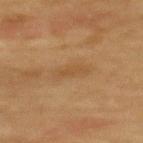automated metrics: a footprint of about 3.5 mm², a shape eccentricity near 0.95, and a shape-asymmetry score of about 0.25 (0 = symmetric); an average lesion color of about L≈43 a*≈16 b*≈33 (CIELAB) and a normalized lesion–skin contrast near 5; a border-irregularity rating of about 3.5/10, internal color variation of about 0.5 on a 0–10 scale, and radial color variation of about 0; an automated nevus-likeness rating near 0 out of 100 and a lesion-detection confidence of about 100/100
patient: female, approximately 55 years of age
image source: ~15 mm tile from a whole-body skin photo
location: the upper back
diameter: about 3.5 mm